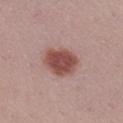biopsy status — catalogued during a skin exam; not biopsied | subject — female, aged 23 to 27 | acquisition — 15 mm crop, total-body photography | illumination — white-light illumination | size — ≈4.5 mm | location — the leg.